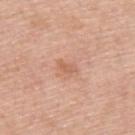Findings:
* notes — total-body-photography surveillance lesion; no biopsy
* image-analysis metrics — an outline eccentricity of about 0.8 (0 = round, 1 = elongated) and two-axis asymmetry of about 0.35; a nevus-likeness score of about 0/100 and a detector confidence of about 100 out of 100 that the crop contains a lesion
* subject — male, approximately 50 years of age
* tile lighting — white-light illumination
* location — the upper back
* lesion diameter — ~2.5 mm (longest diameter)
* image source — 15 mm crop, total-body photography